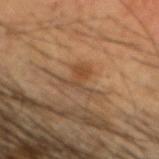Assessment: The lesion was photographed on a routine skin check and not biopsied; there is no pathology result. Clinical summary: A 15 mm close-up extracted from a 3D total-body photography capture. The tile uses cross-polarized illumination. Longest diameter approximately 4 mm. A male subject aged 53–57. Automated image analysis of the tile measured two-axis asymmetry of about 0.65. And it measured border irregularity of about 9.5 on a 0–10 scale, internal color variation of about 1.5 on a 0–10 scale, and peripheral color asymmetry of about 0.5. And it measured a classifier nevus-likeness of about 0/100. On the head or neck.